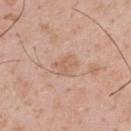Findings:
• illumination · white-light
• lesion size · ≈3 mm
• image · 15 mm crop, total-body photography
• body site · the upper back
• patient · male, about 55 years old
• automated metrics · an area of roughly 5 mm², an outline eccentricity of about 0.6 (0 = round, 1 = elongated), and a shape-asymmetry score of about 0.25 (0 = symmetric); a lesion color around L≈61 a*≈20 b*≈30 in CIELAB, a lesion–skin lightness drop of about 7, and a normalized border contrast of about 5